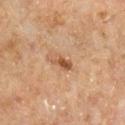No biopsy was performed on this lesion — it was imaged during a full skin examination and was not determined to be concerning. Located on the left lower leg. Cropped from a total-body skin-imaging series; the visible field is about 15 mm. The subject is a male in their mid-60s. Captured under cross-polarized illumination.